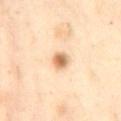biopsy status: no biopsy performed (imaged during a skin exam) | subject: female, aged approximately 55 | lighting: cross-polarized | anatomic site: the abdomen | TBP lesion metrics: an area of roughly 4 mm², an outline eccentricity of about 0.65 (0 = round, 1 = elongated), and two-axis asymmetry of about 0.25; roughly 14 lightness units darker than nearby skin and a lesion-to-skin contrast of about 9 (normalized; higher = more distinct) | image source: total-body-photography crop, ~15 mm field of view | diameter: ~2.5 mm (longest diameter).